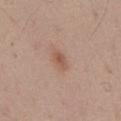biopsy_status: not biopsied; imaged during a skin examination
lighting: white-light
automated_metrics:
  shape_asymmetry: 0.3
  border_irregularity_0_10: 2.5
  color_variation_0_10: 2.0
  peripheral_color_asymmetry: 1.0
  nevus_likeness_0_100: 65
  lesion_detection_confidence_0_100: 100
lesion_size:
  long_diameter_mm_approx: 2.5
patient:
  sex: male
  age_approx: 60
image:
  source: total-body photography crop
  field_of_view_mm: 15
site: chest